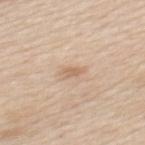Part of a total-body skin-imaging series; this lesion was reviewed on a skin check and was not flagged for biopsy. Measured at roughly 2.5 mm in maximum diameter. The patient is a male aged 58 to 62. On the mid back. Captured under white-light illumination. Cropped from a total-body skin-imaging series; the visible field is about 15 mm. The lesion-visualizer software estimated border irregularity of about 2.5 on a 0–10 scale and internal color variation of about 1.5 on a 0–10 scale. It also reported an automated nevus-likeness rating near 0 out of 100 and a detector confidence of about 100 out of 100 that the crop contains a lesion.